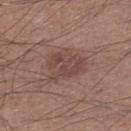Imaged during a routine full-body skin examination; the lesion was not biopsied and no histopathology is available. The lesion is on the right lower leg. Cropped from a total-body skin-imaging series; the visible field is about 15 mm. The patient is a male aged 48–52. Captured under white-light illumination. The lesion-visualizer software estimated a lesion color around L≈45 a*≈19 b*≈21 in CIELAB and a normalized border contrast of about 6. It also reported a border-irregularity index near 4/10, a color-variation rating of about 3/10, and peripheral color asymmetry of about 1. About 5 mm across.